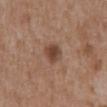Assessment: Imaged during a routine full-body skin examination; the lesion was not biopsied and no histopathology is available. Context: A 15 mm close-up extracted from a 3D total-body photography capture. From the abdomen. Measured at roughly 2.5 mm in maximum diameter. This is a white-light tile. Automated image analysis of the tile measured an area of roughly 5 mm² and an eccentricity of roughly 0.55. The software also gave an average lesion color of about L≈44 a*≈19 b*≈27 (CIELAB), about 11 CIELAB-L* units darker than the surrounding skin, and a normalized lesion–skin contrast near 8.5. A male patient, in their mid- to late 70s.